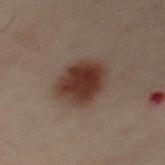Q: Was this lesion biopsied?
A: catalogued during a skin exam; not biopsied
Q: Patient demographics?
A: male, about 50 years old
Q: Where on the body is the lesion?
A: the chest
Q: What kind of image is this?
A: 15 mm crop, total-body photography
Q: Illumination type?
A: cross-polarized